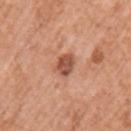{
  "biopsy_status": "not biopsied; imaged during a skin examination",
  "automated_metrics": {
    "area_mm2_approx": 5.0,
    "eccentricity": 0.6,
    "shape_asymmetry": 0.25,
    "cielab_L": 52,
    "cielab_a": 26,
    "cielab_b": 32,
    "vs_skin_darker_L": 13.0,
    "vs_skin_contrast_norm": 9.0,
    "color_variation_0_10": 2.5,
    "peripheral_color_asymmetry": 1.0
  },
  "image": {
    "source": "total-body photography crop",
    "field_of_view_mm": 15
  },
  "patient": {
    "sex": "female",
    "age_approx": 40
  },
  "lesion_size": {
    "long_diameter_mm_approx": 3.0
  },
  "site": "left upper arm"
}